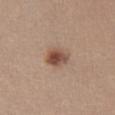This lesion was catalogued during total-body skin photography and was not selected for biopsy.